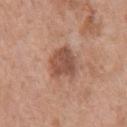<lesion>
<biopsy_status>not biopsied; imaged during a skin examination</biopsy_status>
<lighting>white-light</lighting>
<automated_metrics>
  <area_mm2_approx>11.0</area_mm2_approx>
  <eccentricity>0.55</eccentricity>
  <shape_asymmetry>0.25</shape_asymmetry>
  <cielab_L>51</cielab_L>
  <cielab_a>22</cielab_a>
  <cielab_b>28</cielab_b>
  <vs_skin_darker_L>12.0</vs_skin_darker_L>
  <vs_skin_contrast_norm>8.0</vs_skin_contrast_norm>
  <border_irregularity_0_10>2.5</border_irregularity_0_10>
  <color_variation_0_10>3.5</color_variation_0_10>
  <peripheral_color_asymmetry>1.0</peripheral_color_asymmetry>
</automated_metrics>
<lesion_size>
  <long_diameter_mm_approx>4.0</long_diameter_mm_approx>
</lesion_size>
<patient>
  <sex>male</sex>
  <age_approx>70</age_approx>
</patient>
<site>front of the torso</site>
<image>
  <source>total-body photography crop</source>
  <field_of_view_mm>15</field_of_view_mm>
</image>
</lesion>